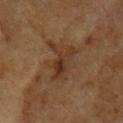Q: Was a biopsy performed?
A: imaged on a skin check; not biopsied
Q: Patient demographics?
A: female, approximately 60 years of age
Q: What is the anatomic site?
A: the head or neck
Q: How was this image acquired?
A: ~15 mm tile from a whole-body skin photo
Q: Lesion size?
A: about 5 mm
Q: What lighting was used for the tile?
A: cross-polarized